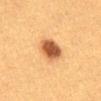The lesion was photographed on a routine skin check and not biopsied; there is no pathology result. A female subject, aged 38 to 42. On the abdomen. The total-body-photography lesion software estimated an area of roughly 9 mm² and two-axis asymmetry of about 0.1. The software also gave internal color variation of about 4.5 on a 0–10 scale and radial color variation of about 1. A 15 mm crop from a total-body photograph taken for skin-cancer surveillance.